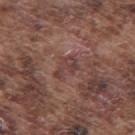Captured during whole-body skin photography for melanoma surveillance; the lesion was not biopsied. Cropped from a total-body skin-imaging series; the visible field is about 15 mm. On the back. A male subject aged approximately 75.